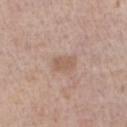Clinical impression: No biopsy was performed on this lesion — it was imaged during a full skin examination and was not determined to be concerning. Clinical summary: The lesion is located on the right forearm. Approximately 2.5 mm at its widest. Automated image analysis of the tile measured an average lesion color of about L≈57 a*≈18 b*≈28 (CIELAB), a lesion–skin lightness drop of about 8, and a normalized border contrast of about 5.5. It also reported a border-irregularity index near 2/10. A lesion tile, about 15 mm wide, cut from a 3D total-body photograph. A male patient, roughly 60 years of age.